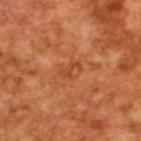Notes:
– workup · imaged on a skin check; not biopsied
– automated metrics · a within-lesion color-variation index near 0/10
– imaging modality · 15 mm crop, total-body photography
– illumination · cross-polarized
– patient · male, aged approximately 65
– lesion size · ~2.5 mm (longest diameter)